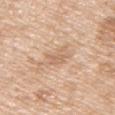This lesion was catalogued during total-body skin photography and was not selected for biopsy. A male patient, in their mid- to late 60s. Approximately 2.5 mm at its widest. A 15 mm close-up extracted from a 3D total-body photography capture. This is a white-light tile. Located on the right upper arm.